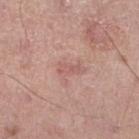Context:
From the leg. The total-body-photography lesion software estimated an area of roughly 2.5 mm² and a shape eccentricity near 0.95. And it measured a border-irregularity rating of about 4/10, internal color variation of about 0 on a 0–10 scale, and radial color variation of about 0. It also reported a classifier nevus-likeness of about 0/100 and a lesion-detection confidence of about 100/100. The tile uses white-light illumination. Cropped from a total-body skin-imaging series; the visible field is about 15 mm. A male patient approximately 55 years of age.